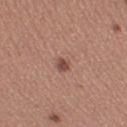biopsy status = no biopsy performed (imaged during a skin exam) | image = total-body-photography crop, ~15 mm field of view | tile lighting = white-light | image-analysis metrics = a lesion color around L≈52 a*≈20 b*≈25 in CIELAB, about 7 CIELAB-L* units darker than the surrounding skin, and a normalized lesion–skin contrast near 5 | subject = female, aged 28 to 32 | location = the right thigh.